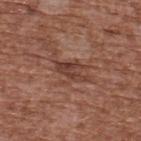Captured during whole-body skin photography for melanoma surveillance; the lesion was not biopsied. A lesion tile, about 15 mm wide, cut from a 3D total-body photograph. Automated image analysis of the tile measured a detector confidence of about 70 out of 100 that the crop contains a lesion. Imaged with white-light lighting. Approximately 4 mm at its widest. A male subject approximately 75 years of age. The lesion is on the upper back.